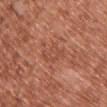Q: Was this lesion biopsied?
A: imaged on a skin check; not biopsied
Q: How was the tile lit?
A: white-light
Q: What is the anatomic site?
A: the chest
Q: Lesion size?
A: about 4 mm
Q: Patient demographics?
A: female, aged approximately 40
Q: What is the imaging modality?
A: total-body-photography crop, ~15 mm field of view
Q: What did automated image analysis measure?
A: an eccentricity of roughly 0.85 and a symmetry-axis asymmetry near 0.3; an average lesion color of about L≈50 a*≈26 b*≈32 (CIELAB), roughly 6 lightness units darker than nearby skin, and a lesion-to-skin contrast of about 4.5 (normalized; higher = more distinct)